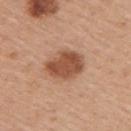workup: no biopsy performed (imaged during a skin exam)
lesion size: about 5 mm
TBP lesion metrics: a lesion area of about 13 mm² and a shape-asymmetry score of about 0.15 (0 = symmetric); a lesion color around L≈52 a*≈23 b*≈33 in CIELAB, about 13 CIELAB-L* units darker than the surrounding skin, and a normalized lesion–skin contrast near 9; internal color variation of about 3 on a 0–10 scale and peripheral color asymmetry of about 1; a classifier nevus-likeness of about 95/100 and lesion-presence confidence of about 100/100
illumination: white-light
patient: male, approximately 55 years of age
location: the upper back
image: ~15 mm tile from a whole-body skin photo